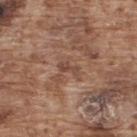<lesion>
  <biopsy_status>not biopsied; imaged during a skin examination</biopsy_status>
  <site>upper back</site>
  <automated_metrics>
    <border_irregularity_0_10>4.0</border_irregularity_0_10>
    <color_variation_0_10>0.5</color_variation_0_10>
    <peripheral_color_asymmetry>0.0</peripheral_color_asymmetry>
  </automated_metrics>
  <lesion_size>
    <long_diameter_mm_approx>2.5</long_diameter_mm_approx>
  </lesion_size>
  <lighting>white-light</lighting>
  <image>
    <source>total-body photography crop</source>
    <field_of_view_mm>15</field_of_view_mm>
  </image>
  <patient>
    <sex>male</sex>
    <age_approx>75</age_approx>
  </patient>
</lesion>